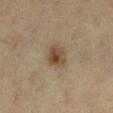<case>
  <biopsy_status>not biopsied; imaged during a skin examination</biopsy_status>
  <site>leg</site>
  <lighting>cross-polarized</lighting>
  <lesion_size>
    <long_diameter_mm_approx>3.0</long_diameter_mm_approx>
  </lesion_size>
  <image>
    <source>total-body photography crop</source>
    <field_of_view_mm>15</field_of_view_mm>
  </image>
  <patient>
    <sex>female</sex>
    <age_approx>55</age_approx>
  </patient>
</case>